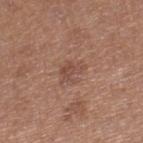This lesion was catalogued during total-body skin photography and was not selected for biopsy.
The subject is a female aged 38–42.
The tile uses white-light illumination.
A 15 mm close-up extracted from a 3D total-body photography capture.
About 2.5 mm across.
From the right lower leg.
The lesion-visualizer software estimated an area of roughly 4.5 mm² and a shape-asymmetry score of about 0.35 (0 = symmetric). The analysis additionally found a nevus-likeness score of about 0/100 and a lesion-detection confidence of about 100/100.